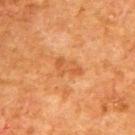Impression: Recorded during total-body skin imaging; not selected for excision or biopsy. Context: The lesion is on the upper back. The patient is a male aged 78 to 82. Longest diameter approximately 3.5 mm. The lesion-visualizer software estimated an outline eccentricity of about 0.8 (0 = round, 1 = elongated) and a symmetry-axis asymmetry near 0.35. The analysis additionally found border irregularity of about 3.5 on a 0–10 scale, internal color variation of about 2.5 on a 0–10 scale, and peripheral color asymmetry of about 1. Captured under cross-polarized illumination. Cropped from a whole-body photographic skin survey; the tile spans about 15 mm.